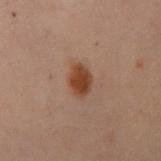Case summary:
- workup · total-body-photography surveillance lesion; no biopsy
- tile lighting · cross-polarized illumination
- subject · female, approximately 30 years of age
- image · ~15 mm tile from a whole-body skin photo
- diameter · ≈3 mm
- site · the left arm